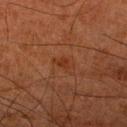This lesion was catalogued during total-body skin photography and was not selected for biopsy.
Located on the right lower leg.
The tile uses cross-polarized illumination.
Cropped from a whole-body photographic skin survey; the tile spans about 15 mm.
A male patient, in their 80s.
Longest diameter approximately 2.5 mm.
Automated tile analysis of the lesion measured a footprint of about 3 mm², a shape eccentricity near 0.8, and a symmetry-axis asymmetry near 0.3. It also reported an average lesion color of about L≈27 a*≈20 b*≈28 (CIELAB) and roughly 5 lightness units darker than nearby skin. The analysis additionally found a border-irregularity rating of about 3/10, a color-variation rating of about 0.5/10, and peripheral color asymmetry of about 0. The analysis additionally found a nevus-likeness score of about 0/100 and lesion-presence confidence of about 100/100.